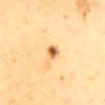patient — female, aged 53 to 57 | automated lesion analysis — a footprint of about 3 mm², an eccentricity of roughly 0.75, and a symmetry-axis asymmetry near 0.2; a lesion color around L≈68 a*≈23 b*≈47 in CIELAB and a lesion–skin lightness drop of about 18; an automated nevus-likeness rating near 100 out of 100 and a lesion-detection confidence of about 100/100 | lesion size — about 2 mm | tile lighting — cross-polarized illumination | image source — total-body-photography crop, ~15 mm field of view | anatomic site — the mid back.